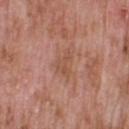The lesion was photographed on a routine skin check and not biopsied; there is no pathology result. On the back. A male patient, in their 60s. A 15 mm crop from a total-body photograph taken for skin-cancer surveillance.